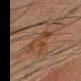{
  "biopsy_status": "not biopsied; imaged during a skin examination",
  "image": {
    "source": "total-body photography crop",
    "field_of_view_mm": 15
  },
  "site": "head or neck",
  "lesion_size": {
    "long_diameter_mm_approx": 5.0
  },
  "patient": {
    "sex": "female",
    "age_approx": 60
  }
}